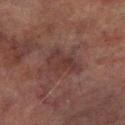{"biopsy_status": "not biopsied; imaged during a skin examination", "site": "right lower leg", "image": {"source": "total-body photography crop", "field_of_view_mm": 15}, "patient": {"sex": "male", "age_approx": 75}, "lesion_size": {"long_diameter_mm_approx": 4.5}, "lighting": "cross-polarized"}